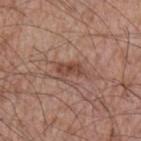workup: no biopsy performed (imaged during a skin exam)
lighting: white-light
patient: male, approximately 65 years of age
image: total-body-photography crop, ~15 mm field of view
image-analysis metrics: a lesion area of about 5.5 mm², a shape eccentricity near 0.85, and a symmetry-axis asymmetry near 0.4; a border-irregularity rating of about 5/10 and peripheral color asymmetry of about 1; a nevus-likeness score of about 10/100 and a detector confidence of about 100 out of 100 that the crop contains a lesion
lesion size: ~3.5 mm (longest diameter)
site: the arm